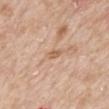Clinical impression:
Imaged during a routine full-body skin examination; the lesion was not biopsied and no histopathology is available.
Background:
The lesion-visualizer software estimated a lesion area of about 2.5 mm², an eccentricity of roughly 0.85, and a shape-asymmetry score of about 0.35 (0 = symmetric). And it measured a lesion color around L≈62 a*≈20 b*≈33 in CIELAB, about 8 CIELAB-L* units darker than the surrounding skin, and a normalized border contrast of about 6. It also reported border irregularity of about 3 on a 0–10 scale, a within-lesion color-variation index near 2.5/10, and radial color variation of about 1. And it measured a classifier nevus-likeness of about 0/100 and a lesion-detection confidence of about 100/100. This is a white-light tile. Longest diameter approximately 2.5 mm. The lesion is on the mid back. This image is a 15 mm lesion crop taken from a total-body photograph. A male patient aged 63–67.